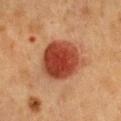Part of a total-body skin-imaging series; this lesion was reviewed on a skin check and was not flagged for biopsy. The subject is a female aged 38 to 42. The lesion's longest dimension is about 5.5 mm. Imaged with cross-polarized lighting. A roughly 15 mm field-of-view crop from a total-body skin photograph. The lesion is located on the chest. The lesion-visualizer software estimated a footprint of about 22 mm², an outline eccentricity of about 0.35 (0 = round, 1 = elongated), and a shape-asymmetry score of about 0.15 (0 = symmetric). The analysis additionally found an average lesion color of about L≈39 a*≈28 b*≈31 (CIELAB), about 15 CIELAB-L* units darker than the surrounding skin, and a normalized border contrast of about 11.5. It also reported a border-irregularity rating of about 1.5/10 and internal color variation of about 5 on a 0–10 scale.